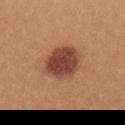Imaged during a routine full-body skin examination; the lesion was not biopsied and no histopathology is available. A 15 mm close-up tile from a total-body photography series done for melanoma screening. Approximately 4.5 mm at its widest. A female subject, aged 23 to 27. From the left upper arm.